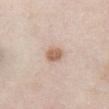biopsy status = total-body-photography surveillance lesion; no biopsy | subject = female, about 60 years old | lesion diameter = about 3 mm | location = the abdomen | image source = 15 mm crop, total-body photography | lighting = white-light.